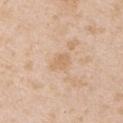Impression:
Recorded during total-body skin imaging; not selected for excision or biopsy.
Clinical summary:
Measured at roughly 2.5 mm in maximum diameter. The patient is a male aged 43 to 47. A 15 mm close-up extracted from a 3D total-body photography capture. The lesion is on the left upper arm.